A lesion tile, about 15 mm wide, cut from a 3D total-body photograph. The patient is a male aged 43–47. The recorded lesion diameter is about 5.5 mm. The lesion is located on the upper back.
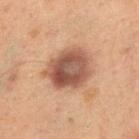diagnosis = a melanocytic nevus.